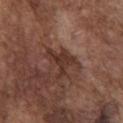Part of a total-body skin-imaging series; this lesion was reviewed on a skin check and was not flagged for biopsy. A region of skin cropped from a whole-body photographic capture, roughly 15 mm wide. A male patient in their mid- to late 70s. The lesion is on the chest.